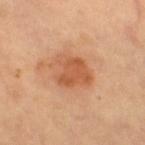Assessment:
Captured during whole-body skin photography for melanoma surveillance; the lesion was not biopsied.
Context:
Automated image analysis of the tile measured a border-irregularity rating of about 2.5/10, a within-lesion color-variation index near 3.5/10, and a peripheral color-asymmetry measure near 1. The lesion is on the right thigh. This is a cross-polarized tile. This image is a 15 mm lesion crop taken from a total-body photograph. A subject about 60 years old. Longest diameter approximately 4 mm.